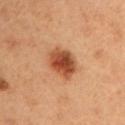Notes:
* notes · no biopsy performed (imaged during a skin exam)